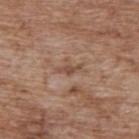follow-up = no biopsy performed (imaged during a skin exam); subject = male, aged approximately 70; image = ~15 mm tile from a whole-body skin photo; anatomic site = the back.